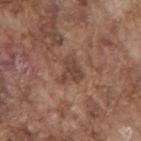Recorded during total-body skin imaging; not selected for excision or biopsy. A roughly 15 mm field-of-view crop from a total-body skin photograph. Imaged with white-light lighting. Located on the mid back. An algorithmic analysis of the crop reported a lesion–skin lightness drop of about 8. And it measured a border-irregularity index near 5.5/10 and a peripheral color-asymmetry measure near 1. And it measured a classifier nevus-likeness of about 10/100 and a lesion-detection confidence of about 100/100. A male subject in their mid- to late 70s. About 3.5 mm across.